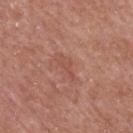Impression:
Captured during whole-body skin photography for melanoma surveillance; the lesion was not biopsied.
Image and clinical context:
A lesion tile, about 15 mm wide, cut from a 3D total-body photograph. From the mid back. The subject is a male in their mid- to late 60s.